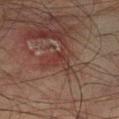Findings:
* workup: imaged on a skin check; not biopsied
* anatomic site: the left lower leg
* patient: male, approximately 75 years of age
* image source: ~15 mm crop, total-body skin-cancer survey